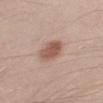biopsy status: total-body-photography surveillance lesion; no biopsy
location: the arm
image-analysis metrics: an area of roughly 7.5 mm², a shape eccentricity near 0.8, and a symmetry-axis asymmetry near 0.2; a lesion–skin lightness drop of about 12 and a normalized lesion–skin contrast near 8
subject: male, aged around 40
imaging modality: 15 mm crop, total-body photography
lesion size: ≈4 mm